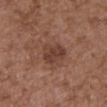Assessment: Captured during whole-body skin photography for melanoma surveillance; the lesion was not biopsied. Background: Measured at roughly 3.5 mm in maximum diameter. The lesion-visualizer software estimated a lesion–skin lightness drop of about 9 and a normalized border contrast of about 7.5. And it measured border irregularity of about 2.5 on a 0–10 scale, a color-variation rating of about 3/10, and a peripheral color-asymmetry measure near 1. The analysis additionally found a classifier nevus-likeness of about 0/100 and a detector confidence of about 100 out of 100 that the crop contains a lesion. A 15 mm close-up tile from a total-body photography series done for melanoma screening. A male subject, in their mid-70s. On the abdomen.